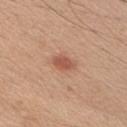Q: Was this lesion biopsied?
A: catalogued during a skin exam; not biopsied
Q: Lesion location?
A: the chest
Q: What kind of image is this?
A: ~15 mm tile from a whole-body skin photo
Q: What lighting was used for the tile?
A: white-light
Q: What are the patient's age and sex?
A: male, in their 20s